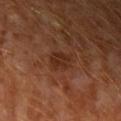{"biopsy_status": "not biopsied; imaged during a skin examination", "patient": {"sex": "male", "age_approx": 60}, "lighting": "cross-polarized", "automated_metrics": {"cielab_L": 27, "cielab_a": 21, "cielab_b": 27, "vs_skin_darker_L": 6.0, "vs_skin_contrast_norm": 7.0}, "site": "left upper arm", "image": {"source": "total-body photography crop", "field_of_view_mm": 15}}